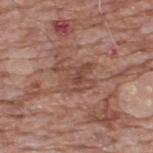{"biopsy_status": "not biopsied; imaged during a skin examination", "lighting": "white-light", "patient": {"sex": "male", "age_approx": 60}, "image": {"source": "total-body photography crop", "field_of_view_mm": 15}, "lesion_size": {"long_diameter_mm_approx": 4.0}, "automated_metrics": {"area_mm2_approx": 8.5, "eccentricity": 0.65, "shape_asymmetry": 0.5, "cielab_L": 46, "cielab_a": 22, "cielab_b": 27, "vs_skin_darker_L": 8.0, "vs_skin_contrast_norm": 6.0}, "site": "upper back"}